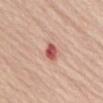Impression: The lesion was tiled from a total-body skin photograph and was not biopsied.